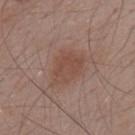Findings:
- site · the back
- subject · male, aged approximately 55
- imaging modality · total-body-photography crop, ~15 mm field of view
- diameter · ~4 mm (longest diameter)